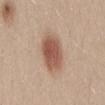subject: female, aged around 30 | anatomic site: the mid back | automated lesion analysis: a footprint of about 13 mm², an eccentricity of roughly 0.65, and a shape-asymmetry score of about 0.15 (0 = symmetric); a border-irregularity rating of about 1.5/10, internal color variation of about 3.5 on a 0–10 scale, and peripheral color asymmetry of about 1 | acquisition: total-body-photography crop, ~15 mm field of view | lighting: white-light | lesion diameter: about 4.5 mm.